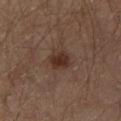workup: total-body-photography surveillance lesion; no biopsy | patient: male, aged approximately 65 | body site: the leg | size: ≈2.5 mm | image: ~15 mm crop, total-body skin-cancer survey | illumination: cross-polarized.